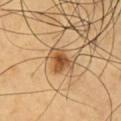- workup: total-body-photography surveillance lesion; no biopsy
- location: the front of the torso
- illumination: cross-polarized illumination
- image: ~15 mm tile from a whole-body skin photo
- subject: male, roughly 60 years of age
- lesion diameter: about 4 mm